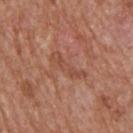Notes:
• notes · no biopsy performed (imaged during a skin exam)
• lesion size · ≈4.5 mm
• site · the upper back
• subject · male, aged 63–67
• automated lesion analysis · a lesion area of about 6 mm², an outline eccentricity of about 0.95 (0 = round, 1 = elongated), and a symmetry-axis asymmetry near 0.35; roughly 7 lightness units darker than nearby skin and a lesion-to-skin contrast of about 5 (normalized; higher = more distinct); a border-irregularity rating of about 5.5/10
• tile lighting · white-light illumination
• imaging modality · total-body-photography crop, ~15 mm field of view